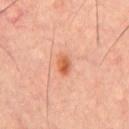Recorded during total-body skin imaging; not selected for excision or biopsy.
From the chest.
A male patient aged approximately 45.
The lesion's longest dimension is about 2.5 mm.
The lesion-visualizer software estimated a footprint of about 4 mm² and an outline eccentricity of about 0.75 (0 = round, 1 = elongated). The software also gave a lesion color around L≈58 a*≈27 b*≈37 in CIELAB, roughly 10 lightness units darker than nearby skin, and a normalized border contrast of about 8. The analysis additionally found an automated nevus-likeness rating near 95 out of 100 and a lesion-detection confidence of about 100/100.
Captured under cross-polarized illumination.
Cropped from a whole-body photographic skin survey; the tile spans about 15 mm.